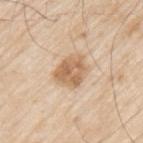Impression:
Recorded during total-body skin imaging; not selected for excision or biopsy.
Image and clinical context:
The patient is a male in their 80s. The lesion is on the left upper arm. The total-body-photography lesion software estimated internal color variation of about 4.5 on a 0–10 scale and peripheral color asymmetry of about 2. This image is a 15 mm lesion crop taken from a total-body photograph. Measured at roughly 4 mm in maximum diameter.